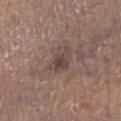Impression:
The lesion was tiled from a total-body skin photograph and was not biopsied.
Context:
Located on the leg. A male subject aged 73 to 77. A 15 mm crop from a total-body photograph taken for skin-cancer surveillance. Measured at roughly 3.5 mm in maximum diameter.